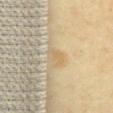| field | value |
|---|---|
| notes | no biopsy performed (imaged during a skin exam) |
| location | the chest |
| automated lesion analysis | an eccentricity of roughly 0.9 and two-axis asymmetry of about 0.4; border irregularity of about 4 on a 0–10 scale, internal color variation of about 0 on a 0–10 scale, and radial color variation of about 0; an automated nevus-likeness rating near 0 out of 100 and a detector confidence of about 100 out of 100 that the crop contains a lesion |
| imaging modality | 15 mm crop, total-body photography |
| lighting | cross-polarized |
| patient | female, in their mid- to late 50s |
| lesion size | ≈2.5 mm |